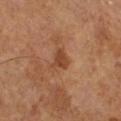The patient is a male aged approximately 65.
A lesion tile, about 15 mm wide, cut from a 3D total-body photograph.
Measured at roughly 3 mm in maximum diameter.
The lesion is located on the left leg.
The tile uses cross-polarized illumination.